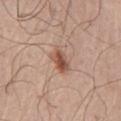Part of a total-body skin-imaging series; this lesion was reviewed on a skin check and was not flagged for biopsy.